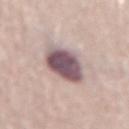Clinical impression: Captured during whole-body skin photography for melanoma surveillance; the lesion was not biopsied. Background: The patient is a female in their mid- to late 60s. A 15 mm close-up tile from a total-body photography series done for melanoma screening. Captured under white-light illumination. The recorded lesion diameter is about 4.5 mm. Located on the lower back.